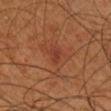No biopsy was performed on this lesion — it was imaged during a full skin examination and was not determined to be concerning.
A male patient approximately 70 years of age.
An algorithmic analysis of the crop reported a mean CIELAB color near L≈37 a*≈26 b*≈30 and about 6 CIELAB-L* units darker than the surrounding skin.
A 15 mm crop from a total-body photograph taken for skin-cancer surveillance.
Approximately 2.5 mm at its widest.
Imaged with cross-polarized lighting.
The lesion is on the right thigh.